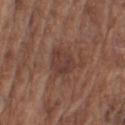follow-up: no biopsy performed (imaged during a skin exam)
image-analysis metrics: a mean CIELAB color near L≈39 a*≈19 b*≈24, roughly 7 lightness units darker than nearby skin, and a normalized border contrast of about 6.5
illumination: white-light illumination
image source: ~15 mm crop, total-body skin-cancer survey
patient: male, in their mid- to late 70s
location: the right upper arm